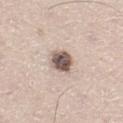Q: Was this lesion biopsied?
A: no biopsy performed (imaged during a skin exam)
Q: Patient demographics?
A: male, aged around 65
Q: Illumination type?
A: white-light
Q: What kind of image is this?
A: ~15 mm tile from a whole-body skin photo
Q: Where on the body is the lesion?
A: the right thigh
Q: What is the lesion's diameter?
A: ~3 mm (longest diameter)
Q: Automated lesion metrics?
A: a lesion color around L≈56 a*≈14 b*≈21 in CIELAB and a lesion–skin lightness drop of about 19; a border-irregularity rating of about 1.5/10, a color-variation rating of about 5.5/10, and radial color variation of about 1.5